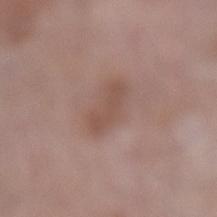<lesion>
<biopsy_status>not biopsied; imaged during a skin examination</biopsy_status>
<patient>
  <sex>female</sex>
  <age_approx>70</age_approx>
</patient>
<image>
  <source>total-body photography crop</source>
  <field_of_view_mm>15</field_of_view_mm>
</image>
<site>left lower leg</site>
</lesion>